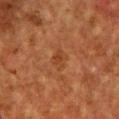- biopsy status — catalogued during a skin exam; not biopsied
- TBP lesion metrics — a footprint of about 3.5 mm², a shape eccentricity near 0.8, and a shape-asymmetry score of about 0.25 (0 = symmetric); a classifier nevus-likeness of about 0/100 and a lesion-detection confidence of about 100/100
- acquisition — ~15 mm crop, total-body skin-cancer survey
- location — the chest
- diameter — about 2.5 mm
- tile lighting — cross-polarized
- patient — male, aged 58–62